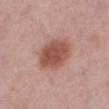This lesion was catalogued during total-body skin photography and was not selected for biopsy. A female subject in their 50s. A roughly 15 mm field-of-view crop from a total-body skin photograph. The lesion's longest dimension is about 5 mm. This is a white-light tile. The lesion is on the left lower leg.